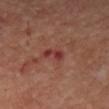- notes: imaged on a skin check; not biopsied
- subject: female, aged approximately 65
- body site: the right forearm
- imaging modality: ~15 mm tile from a whole-body skin photo